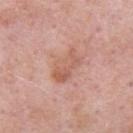Captured during whole-body skin photography for melanoma surveillance; the lesion was not biopsied. The lesion is located on the chest. A male patient, aged approximately 55. This image is a 15 mm lesion crop taken from a total-body photograph.